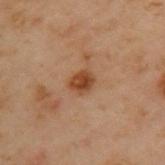Assessment: No biopsy was performed on this lesion — it was imaged during a full skin examination and was not determined to be concerning. Acquisition and patient details: A male subject, approximately 55 years of age. A lesion tile, about 15 mm wide, cut from a 3D total-body photograph. The tile uses cross-polarized illumination. From the upper back. Approximately 2.5 mm at its widest.